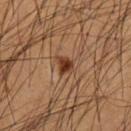Captured during whole-body skin photography for melanoma surveillance; the lesion was not biopsied.
From the front of the torso.
Captured under cross-polarized illumination.
Cropped from a whole-body photographic skin survey; the tile spans about 15 mm.
A male subject approximately 55 years of age.
The total-body-photography lesion software estimated a lesion area of about 3.5 mm², an outline eccentricity of about 0.6 (0 = round, 1 = elongated), and two-axis asymmetry of about 0.45. The analysis additionally found a border-irregularity rating of about 4/10, a color-variation rating of about 3.5/10, and a peripheral color-asymmetry measure near 1.5. The software also gave lesion-presence confidence of about 100/100.
Measured at roughly 2.5 mm in maximum diameter.